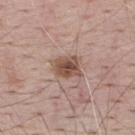{
  "biopsy_status": "not biopsied; imaged during a skin examination"
}